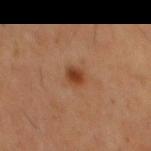Case summary:
- workup: catalogued during a skin exam; not biopsied
- lesion size: about 2 mm
- acquisition: ~15 mm tile from a whole-body skin photo
- location: the back
- subject: male, aged 58–62
- illumination: cross-polarized